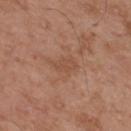Imaged during a routine full-body skin examination; the lesion was not biopsied and no histopathology is available.
This is a white-light tile.
The lesion is located on the back.
A 15 mm crop from a total-body photograph taken for skin-cancer surveillance.
The subject is a male in their mid-50s.